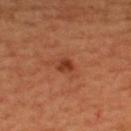Q: What are the patient's age and sex?
A: male, aged around 65
Q: What is the anatomic site?
A: the back
Q: How was this image acquired?
A: total-body-photography crop, ~15 mm field of view